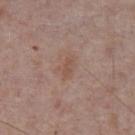notes = imaged on a skin check; not biopsied
patient = male, about 75 years old
imaging modality = total-body-photography crop, ~15 mm field of view
lesion diameter = ≈3 mm
tile lighting = white-light illumination
body site = the chest